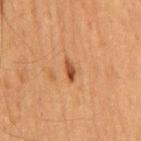notes: no biopsy performed (imaged during a skin exam)
size: ≈2.5 mm
subject: male, aged around 65
site: the chest
image source: total-body-photography crop, ~15 mm field of view
tile lighting: cross-polarized illumination